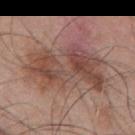<tbp_lesion>
<biopsy_status>not biopsied; imaged during a skin examination</biopsy_status>
<image>
  <source>total-body photography crop</source>
  <field_of_view_mm>15</field_of_view_mm>
</image>
<patient>
  <sex>male</sex>
  <age_approx>55</age_approx>
</patient>
<site>mid back</site>
</tbp_lesion>